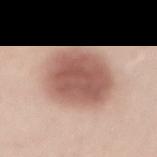{"biopsy_status": "not biopsied; imaged during a skin examination", "lesion_size": {"long_diameter_mm_approx": 7.0}, "image": {"source": "total-body photography crop", "field_of_view_mm": 15}, "patient": {"sex": "male", "age_approx": 60}, "lighting": "white-light", "site": "lower back"}